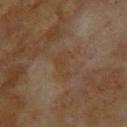| feature | finding |
|---|---|
| biopsy status | total-body-photography surveillance lesion; no biopsy |